Located on the right thigh · the subject is a female roughly 40 years of age · a 15 mm close-up extracted from a 3D total-body photography capture:
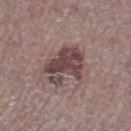| field | value |
|---|---|
| illumination | white-light |
| automated metrics | border irregularity of about 5 on a 0–10 scale, a within-lesion color-variation index near 4.5/10, and radial color variation of about 1.5 |
| pathology | an invasive melanoma; Breslow depth 0.9 mm, mitotic rate 4/mm² |